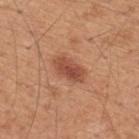Clinical impression:
This lesion was catalogued during total-body skin photography and was not selected for biopsy.
Acquisition and patient details:
Cropped from a whole-body photographic skin survey; the tile spans about 15 mm. Longest diameter approximately 4 mm. On the back. Captured under white-light illumination. A male patient aged 43 to 47. Automated image analysis of the tile measured an area of roughly 7.5 mm², an outline eccentricity of about 0.8 (0 = round, 1 = elongated), and a shape-asymmetry score of about 0.2 (0 = symmetric). The software also gave an average lesion color of about L≈50 a*≈26 b*≈32 (CIELAB) and about 11 CIELAB-L* units darker than the surrounding skin.